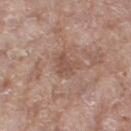A region of skin cropped from a whole-body photographic capture, roughly 15 mm wide.
The lesion is on the leg.
Measured at roughly 3 mm in maximum diameter.
Imaged with white-light lighting.
A female patient in their mid-70s.